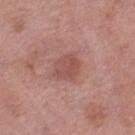Q: Was a biopsy performed?
A: imaged on a skin check; not biopsied
Q: What is the anatomic site?
A: the left thigh
Q: Illumination type?
A: white-light
Q: Patient demographics?
A: female, aged around 70
Q: What is the imaging modality?
A: ~15 mm tile from a whole-body skin photo